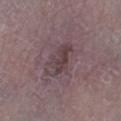Clinical impression: Captured during whole-body skin photography for melanoma surveillance; the lesion was not biopsied.